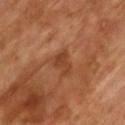biopsy_status: not biopsied; imaged during a skin examination
lesion_size:
  long_diameter_mm_approx: 2.5
lighting: cross-polarized
site: chest
patient:
  sex: male
  age_approx: 65
image:
  source: total-body photography crop
  field_of_view_mm: 15